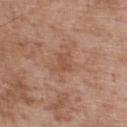Case summary:
* follow-up · no biopsy performed (imaged during a skin exam)
* lighting · white-light
* size · ≈2.5 mm
* body site · the upper back
* patient · male, aged around 50
* image source · total-body-photography crop, ~15 mm field of view
* image-analysis metrics · an area of roughly 4.5 mm² and two-axis asymmetry of about 0.4; an automated nevus-likeness rating near 0 out of 100 and lesion-presence confidence of about 100/100